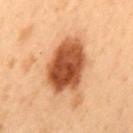| field | value |
|---|---|
| workup | total-body-photography surveillance lesion; no biopsy |
| subject | male, aged 53–57 |
| imaging modality | total-body-photography crop, ~15 mm field of view |
| image-analysis metrics | an area of roughly 24 mm², an eccentricity of roughly 0.7, and a shape-asymmetry score of about 0.15 (0 = symmetric); a mean CIELAB color near L≈43 a*≈23 b*≈33; a border-irregularity index near 1.5/10, a within-lesion color-variation index near 5.5/10, and radial color variation of about 2; lesion-presence confidence of about 100/100 |
| lighting | cross-polarized illumination |
| lesion diameter | ≈6.5 mm |
| body site | the mid back |